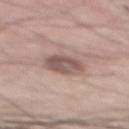Findings:
– notes — total-body-photography surveillance lesion; no biopsy
– image source — 15 mm crop, total-body photography
– location — the back
– patient — male, aged 68–72